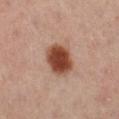follow-up: no biopsy performed (imaged during a skin exam) | acquisition: 15 mm crop, total-body photography | patient: female, aged 38–42 | automated metrics: a footprint of about 12 mm² and a shape-asymmetry score of about 0.15 (0 = symmetric) | lesion size: about 4.5 mm | anatomic site: the right leg.